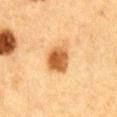Impression: No biopsy was performed on this lesion — it was imaged during a full skin examination and was not determined to be concerning. Background: A male patient, roughly 85 years of age or older. Cropped from a whole-body photographic skin survey; the tile spans about 15 mm. Located on the front of the torso.